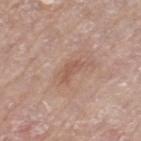Recorded during total-body skin imaging; not selected for excision or biopsy. Cropped from a whole-body photographic skin survey; the tile spans about 15 mm. A female subject aged 73–77. The lesion is located on the left thigh.